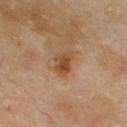Clinical impression: This lesion was catalogued during total-body skin photography and was not selected for biopsy. Clinical summary: The tile uses cross-polarized illumination. A male patient, in their 70s. On the chest. A 15 mm crop from a total-body photograph taken for skin-cancer surveillance. An algorithmic analysis of the crop reported a lesion area of about 5 mm² and two-axis asymmetry of about 0.35. And it measured an average lesion color of about L≈46 a*≈20 b*≈35 (CIELAB), roughly 10 lightness units darker than nearby skin, and a lesion-to-skin contrast of about 8.5 (normalized; higher = more distinct). It also reported a color-variation rating of about 5/10 and radial color variation of about 1.5. About 3 mm across.